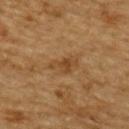Q: Is there a histopathology result?
A: total-body-photography surveillance lesion; no biopsy
Q: How large is the lesion?
A: about 3.5 mm
Q: Where on the body is the lesion?
A: the upper back
Q: Who is the patient?
A: male, aged approximately 85 or older
Q: Automated lesion metrics?
A: a lesion-to-skin contrast of about 6 (normalized; higher = more distinct); border irregularity of about 5 on a 0–10 scale, internal color variation of about 2 on a 0–10 scale, and a peripheral color-asymmetry measure near 0.5; an automated nevus-likeness rating near 5 out of 100 and a lesion-detection confidence of about 100/100
Q: What is the imaging modality?
A: 15 mm crop, total-body photography